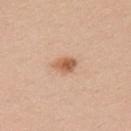Assessment: The lesion was tiled from a total-body skin photograph and was not biopsied. Background: A female patient, in their mid- to late 20s. Longest diameter approximately 3 mm. This is a white-light tile. A 15 mm close-up extracted from a 3D total-body photography capture. The lesion-visualizer software estimated a color-variation rating of about 4/10 and radial color variation of about 1.5. The software also gave an automated nevus-likeness rating near 90 out of 100 and lesion-presence confidence of about 100/100. Located on the left upper arm.